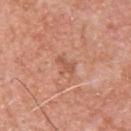No biopsy was performed on this lesion — it was imaged during a full skin examination and was not determined to be concerning. The lesion is on the upper back. This image is a 15 mm lesion crop taken from a total-body photograph. Longest diameter approximately 2.5 mm. The subject is a male in their mid- to late 50s. The tile uses white-light illumination.